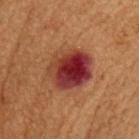Assessment:
Imaged during a routine full-body skin examination; the lesion was not biopsied and no histopathology is available.
Background:
On the head or neck. A male subject about 55 years old. This image is a 15 mm lesion crop taken from a total-body photograph. The total-body-photography lesion software estimated a footprint of about 19 mm², a shape eccentricity near 0.55, and two-axis asymmetry of about 0.15.